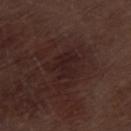  biopsy_status: not biopsied; imaged during a skin examination
  image:
    source: total-body photography crop
    field_of_view_mm: 15
  automated_metrics:
    border_irregularity_0_10: 4.0
    color_variation_0_10: 1.5
    peripheral_color_asymmetry: 0.5
    nevus_likeness_0_100: 0
  patient:
    sex: male
    age_approx: 70
  lighting: white-light
  lesion_size:
    long_diameter_mm_approx: 3.5
  site: left thigh